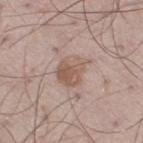follow-up = catalogued during a skin exam; not biopsied | body site = the left thigh | tile lighting = white-light illumination | acquisition = ~15 mm crop, total-body skin-cancer survey | size = about 4 mm | automated metrics = a shape eccentricity near 0.6 and two-axis asymmetry of about 0.3; a lesion color around L≈56 a*≈17 b*≈25 in CIELAB, roughly 9 lightness units darker than nearby skin, and a normalized border contrast of about 7 | subject = male, aged 48 to 52.